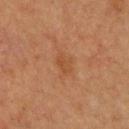Q: Was a biopsy performed?
A: total-body-photography surveillance lesion; no biopsy
Q: What is the anatomic site?
A: the upper back
Q: How was this image acquired?
A: ~15 mm tile from a whole-body skin photo
Q: Patient demographics?
A: male, roughly 60 years of age